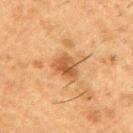notes: catalogued during a skin exam; not biopsied
patient: male, roughly 50 years of age
lighting: cross-polarized illumination
automated lesion analysis: a lesion color around L≈44 a*≈20 b*≈34 in CIELAB and a normalized border contrast of about 7.5; a nevus-likeness score of about 45/100 and a detector confidence of about 100 out of 100 that the crop contains a lesion
diameter: about 3.5 mm
site: the right upper arm
image source: ~15 mm crop, total-body skin-cancer survey